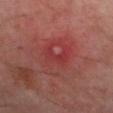Clinical impression: Recorded during total-body skin imaging; not selected for excision or biopsy. Acquisition and patient details: A roughly 15 mm field-of-view crop from a total-body skin photograph. The lesion-visualizer software estimated a lesion area of about 3.5 mm², an eccentricity of roughly 0.9, and two-axis asymmetry of about 0.4. The software also gave a lesion color around L≈35 a*≈33 b*≈23 in CIELAB, a lesion–skin lightness drop of about 5, and a normalized border contrast of about 4.5. The analysis additionally found a color-variation rating of about 0.5/10. Approximately 3 mm at its widest. From the left thigh. A male subject, in their 60s.